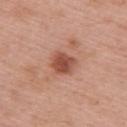Impression:
The lesion was tiled from a total-body skin photograph and was not biopsied.
Acquisition and patient details:
Located on the back. The subject is a male approximately 60 years of age. The lesion's longest dimension is about 3 mm. Captured under white-light illumination. A 15 mm crop from a total-body photograph taken for skin-cancer surveillance. Automated tile analysis of the lesion measured an average lesion color of about L≈50 a*≈26 b*≈30 (CIELAB) and roughly 13 lightness units darker than nearby skin. It also reported a border-irregularity rating of about 2/10 and radial color variation of about 1. The analysis additionally found an automated nevus-likeness rating near 85 out of 100.